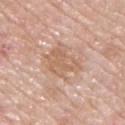Impression:
Part of a total-body skin-imaging series; this lesion was reviewed on a skin check and was not flagged for biopsy.
Background:
Cropped from a total-body skin-imaging series; the visible field is about 15 mm. Longest diameter approximately 5 mm. Automated tile analysis of the lesion measured an average lesion color of about L≈62 a*≈19 b*≈30 (CIELAB), about 8 CIELAB-L* units darker than the surrounding skin, and a normalized lesion–skin contrast near 5.5. It also reported internal color variation of about 3 on a 0–10 scale. The software also gave a classifier nevus-likeness of about 0/100 and a lesion-detection confidence of about 100/100. Captured under white-light illumination. From the chest. A male subject in their mid-50s.